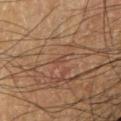Notes:
• image: total-body-photography crop, ~15 mm field of view
• patient: male, in their 60s
• body site: the right forearm
• illumination: cross-polarized illumination
• size: ~1.5 mm (longest diameter)
• histopathology: a nodular basal cell carcinoma (malignant)